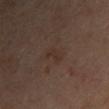<record>
<biopsy_status>not biopsied; imaged during a skin examination</biopsy_status>
<automated_metrics>
  <area_mm2_approx>3.5</area_mm2_approx>
  <shape_asymmetry>0.3</shape_asymmetry>
  <cielab_L>30</cielab_L>
  <cielab_a>15</cielab_a>
  <cielab_b>21</cielab_b>
  <vs_skin_darker_L>4.0</vs_skin_darker_L>
  <vs_skin_contrast_norm>5.0</vs_skin_contrast_norm>
  <nevus_likeness_0_100>0</nevus_likeness_0_100>
  <lesion_detection_confidence_0_100>100</lesion_detection_confidence_0_100>
</automated_metrics>
<image>
  <source>total-body photography crop</source>
  <field_of_view_mm>15</field_of_view_mm>
</image>
<site>right upper arm</site>
<lesion_size>
  <long_diameter_mm_approx>3.0</long_diameter_mm_approx>
</lesion_size>
<patient>
  <sex>female</sex>
  <age_approx>45</age_approx>
</patient>
</record>